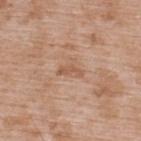Notes:
- follow-up: catalogued during a skin exam; not biopsied
- body site: the back
- tile lighting: white-light illumination
- subject: male, in their 50s
- image source: ~15 mm crop, total-body skin-cancer survey